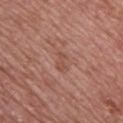Q: Was a biopsy performed?
A: imaged on a skin check; not biopsied
Q: What are the patient's age and sex?
A: male, roughly 50 years of age
Q: What did automated image analysis measure?
A: an average lesion color of about L≈50 a*≈23 b*≈28 (CIELAB) and about 6 CIELAB-L* units darker than the surrounding skin; a classifier nevus-likeness of about 0/100 and lesion-presence confidence of about 100/100
Q: What kind of image is this?
A: ~15 mm tile from a whole-body skin photo
Q: Where on the body is the lesion?
A: the chest
Q: What is the lesion's diameter?
A: ~2.5 mm (longest diameter)
Q: What lighting was used for the tile?
A: white-light